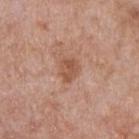Imaged during a routine full-body skin examination; the lesion was not biopsied and no histopathology is available.
Measured at roughly 3 mm in maximum diameter.
A 15 mm close-up tile from a total-body photography series done for melanoma screening.
Automated tile analysis of the lesion measured two-axis asymmetry of about 0.2. It also reported an average lesion color of about L≈53 a*≈22 b*≈31 (CIELAB), about 9 CIELAB-L* units darker than the surrounding skin, and a lesion-to-skin contrast of about 7 (normalized; higher = more distinct). It also reported a border-irregularity index near 2/10 and a within-lesion color-variation index near 2.5/10. The analysis additionally found lesion-presence confidence of about 100/100.
A male patient, approximately 50 years of age.
The lesion is located on the front of the torso.
The tile uses white-light illumination.